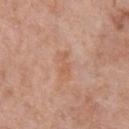• site · the front of the torso
• imaging modality · ~15 mm tile from a whole-body skin photo
• subject · male, in their 70s